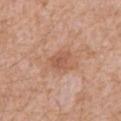Recorded during total-body skin imaging; not selected for excision or biopsy.
On the mid back.
A 15 mm crop from a total-body photograph taken for skin-cancer surveillance.
The subject is a male aged 58 to 62.